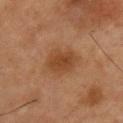Part of a total-body skin-imaging series; this lesion was reviewed on a skin check and was not flagged for biopsy.
Imaged with cross-polarized lighting.
A 15 mm close-up tile from a total-body photography series done for melanoma screening.
Approximately 4 mm at its widest.
A male subject, aged around 55.
On the chest.